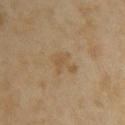Clinical impression: Captured during whole-body skin photography for melanoma surveillance; the lesion was not biopsied. Clinical summary: The lesion is on the upper back. Cropped from a total-body skin-imaging series; the visible field is about 15 mm. The tile uses cross-polarized illumination. A female subject roughly 35 years of age.